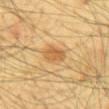Recorded during total-body skin imaging; not selected for excision or biopsy.
A close-up tile cropped from a whole-body skin photograph, about 15 mm across.
The lesion-visualizer software estimated a footprint of about 5 mm², an eccentricity of roughly 0.75, and two-axis asymmetry of about 0.15. It also reported a classifier nevus-likeness of about 90/100 and a detector confidence of about 100 out of 100 that the crop contains a lesion.
The lesion's longest dimension is about 3 mm.
Captured under cross-polarized illumination.
The patient is a male aged 63 to 67.
On the mid back.